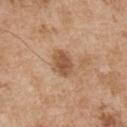<record>
<biopsy_status>not biopsied; imaged during a skin examination</biopsy_status>
<site>right upper arm</site>
<patient>
  <sex>male</sex>
  <age_approx>55</age_approx>
</patient>
<image>
  <source>total-body photography crop</source>
  <field_of_view_mm>15</field_of_view_mm>
</image>
</record>